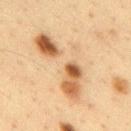{
  "biopsy_status": "not biopsied; imaged during a skin examination",
  "automated_metrics": {
    "cielab_L": 62,
    "cielab_a": 16,
    "cielab_b": 36,
    "vs_skin_darker_L": 10.0,
    "border_irregularity_0_10": 7.5,
    "color_variation_0_10": 10.0
  },
  "site": "back",
  "lighting": "cross-polarized",
  "image": {
    "source": "total-body photography crop",
    "field_of_view_mm": 15
  },
  "patient": {
    "sex": "male",
    "age_approx": 35
  }
}